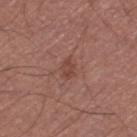notes — imaged on a skin check; not biopsied
imaging modality — ~15 mm tile from a whole-body skin photo
image-analysis metrics — a footprint of about 3 mm² and a shape-asymmetry score of about 0.2 (0 = symmetric); a nevus-likeness score of about 0/100
illumination — white-light illumination
body site — the left thigh
lesion diameter — about 2.5 mm
subject — male, in their mid-60s